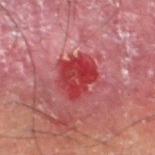Impression:
No biopsy was performed on this lesion — it was imaged during a full skin examination and was not determined to be concerning.
Background:
A close-up tile cropped from a whole-body skin photograph, about 15 mm across. The lesion is located on the left thigh. The subject is a male in their mid-50s.